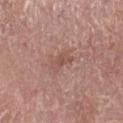{
  "biopsy_status": "not biopsied; imaged during a skin examination",
  "lesion_size": {
    "long_diameter_mm_approx": 3.0
  },
  "lighting": "white-light",
  "image": {
    "source": "total-body photography crop",
    "field_of_view_mm": 15
  },
  "automated_metrics": {
    "area_mm2_approx": 4.0,
    "cielab_L": 51,
    "cielab_a": 21,
    "cielab_b": 24,
    "vs_skin_darker_L": 7.0,
    "vs_skin_contrast_norm": 5.5
  },
  "site": "right lower leg",
  "patient": {
    "sex": "male",
    "age_approx": 75
  }
}